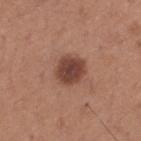{
  "biopsy_status": "not biopsied; imaged during a skin examination",
  "image": {
    "source": "total-body photography crop",
    "field_of_view_mm": 15
  },
  "site": "upper back",
  "lesion_size": {
    "long_diameter_mm_approx": 3.5
  },
  "patient": {
    "sex": "male",
    "age_approx": 65
  },
  "lighting": "white-light"
}